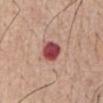follow-up: catalogued during a skin exam; not biopsied
body site: the chest
tile lighting: white-light
size: about 3 mm
image source: ~15 mm crop, total-body skin-cancer survey
patient: male, aged around 55
automated metrics: an eccentricity of roughly 0.6 and a shape-asymmetry score of about 0.15 (0 = symmetric); a lesion color around L≈47 a*≈33 b*≈23 in CIELAB, about 19 CIELAB-L* units darker than the surrounding skin, and a normalized lesion–skin contrast near 13; border irregularity of about 1.5 on a 0–10 scale, internal color variation of about 4 on a 0–10 scale, and a peripheral color-asymmetry measure near 1.5